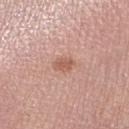This lesion was catalogued during total-body skin photography and was not selected for biopsy.
Located on the arm.
Approximately 2.5 mm at its widest.
A female patient, approximately 50 years of age.
An algorithmic analysis of the crop reported a footprint of about 4 mm² and a shape eccentricity near 0.75. The software also gave a mean CIELAB color near L≈58 a*≈23 b*≈29, a lesion–skin lightness drop of about 9, and a normalized lesion–skin contrast near 6.5. The analysis additionally found border irregularity of about 1.5 on a 0–10 scale and a within-lesion color-variation index near 1.5/10. It also reported an automated nevus-likeness rating near 55 out of 100 and a detector confidence of about 100 out of 100 that the crop contains a lesion.
The tile uses white-light illumination.
Cropped from a whole-body photographic skin survey; the tile spans about 15 mm.